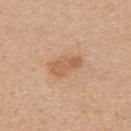  biopsy_status: not biopsied; imaged during a skin examination
  site: upper back
  lighting: white-light
  patient:
    sex: female
    age_approx: 40
  automated_metrics:
    border_irregularity_0_10: 3.5
    color_variation_0_10: 2.5
    peripheral_color_asymmetry: 1.0
  lesion_size:
    long_diameter_mm_approx: 4.0
  image:
    source: total-body photography crop
    field_of_view_mm: 15